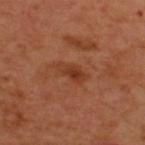imaging modality = 15 mm crop, total-body photography | patient = male, aged approximately 50 | anatomic site = the back | lighting = cross-polarized.